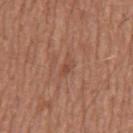The patient is a male about 65 years old. Captured under white-light illumination. Located on the mid back. A 15 mm close-up extracted from a 3D total-body photography capture.